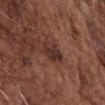{
  "lesion_size": {
    "long_diameter_mm_approx": 3.5
  },
  "patient": {
    "sex": "male",
    "age_approx": 75
  },
  "site": "chest",
  "image": {
    "source": "total-body photography crop",
    "field_of_view_mm": 15
  },
  "automated_metrics": {
    "eccentricity": 0.85,
    "shape_asymmetry": 0.3,
    "cielab_L": 32,
    "cielab_a": 21,
    "cielab_b": 22,
    "vs_skin_darker_L": 9.0
  }
}